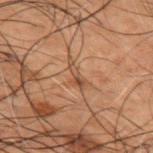Imaged during a routine full-body skin examination; the lesion was not biopsied and no histopathology is available.
About 3 mm across.
On the upper back.
The subject is a male in their 50s.
The lesion-visualizer software estimated a footprint of about 3 mm² and an eccentricity of roughly 0.9. The software also gave a nevus-likeness score of about 0/100 and a lesion-detection confidence of about 60/100.
A 15 mm close-up extracted from a 3D total-body photography capture.
This is a cross-polarized tile.